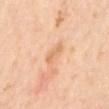<record>
  <biopsy_status>not biopsied; imaged during a skin examination</biopsy_status>
</record>